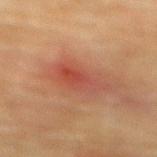Notes:
• notes: total-body-photography surveillance lesion; no biopsy
• image source: ~15 mm tile from a whole-body skin photo
• lesion size: ≈5 mm
• subject: female, aged around 80
• tile lighting: cross-polarized
• image-analysis metrics: an area of roughly 9.5 mm², an eccentricity of roughly 0.85, and a symmetry-axis asymmetry near 0.35; roughly 7 lightness units darker than nearby skin and a normalized border contrast of about 6; a border-irregularity rating of about 3.5/10 and internal color variation of about 5.5 on a 0–10 scale; a nevus-likeness score of about 5/100 and a lesion-detection confidence of about 100/100
• anatomic site: the mid back